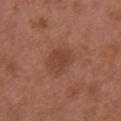subject: female, approximately 65 years of age; imaging modality: ~15 mm tile from a whole-body skin photo; size: about 4.5 mm; body site: the chest; illumination: white-light illumination.